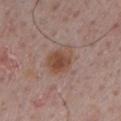Captured during whole-body skin photography for melanoma surveillance; the lesion was not biopsied. Cropped from a whole-body photographic skin survey; the tile spans about 15 mm. A male patient, roughly 55 years of age. Located on the chest. The tile uses white-light illumination. Longest diameter approximately 4 mm.